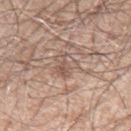{"biopsy_status": "not biopsied; imaged during a skin examination", "patient": {"sex": "male", "age_approx": 60}, "automated_metrics": {"area_mm2_approx": 5.5, "eccentricity": 0.35, "shape_asymmetry": 0.4, "cielab_L": 56, "cielab_a": 17, "cielab_b": 24, "vs_skin_darker_L": 8.0, "nevus_likeness_0_100": 0, "lesion_detection_confidence_0_100": 80}, "lesion_size": {"long_diameter_mm_approx": 3.0}, "site": "left upper arm", "image": {"source": "total-body photography crop", "field_of_view_mm": 15}}